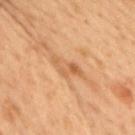workup = catalogued during a skin exam; not biopsied
patient = male, aged 53 to 57
acquisition = 15 mm crop, total-body photography
lighting = cross-polarized
body site = the front of the torso
lesion size = about 4 mm